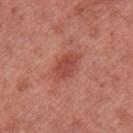biopsy_status: not biopsied; imaged during a skin examination
lighting: white-light
patient:
  sex: female
  age_approx: 50
site: left upper arm
image:
  source: total-body photography crop
  field_of_view_mm: 15
lesion_size:
  long_diameter_mm_approx: 3.5
automated_metrics:
  area_mm2_approx: 6.0
  shape_asymmetry: 0.15
  cielab_L: 48
  cielab_a: 31
  cielab_b: 30
  vs_skin_darker_L: 9.0
  vs_skin_contrast_norm: 6.5
  border_irregularity_0_10: 2.0
  color_variation_0_10: 2.5
  peripheral_color_asymmetry: 1.0
  nevus_likeness_0_100: 80
  lesion_detection_confidence_0_100: 100